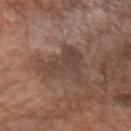<tbp_lesion>
  <biopsy_status>not biopsied; imaged during a skin examination</biopsy_status>
  <lighting>white-light</lighting>
  <site>left forearm</site>
  <image>
    <source>total-body photography crop</source>
    <field_of_view_mm>15</field_of_view_mm>
  </image>
  <lesion_size>
    <long_diameter_mm_approx>6.5</long_diameter_mm_approx>
  </lesion_size>
  <patient>
    <sex>male</sex>
    <age_approx>70</age_approx>
  </patient>
</tbp_lesion>